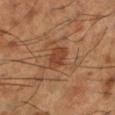A male subject, approximately 65 years of age. A 15 mm crop from a total-body photograph taken for skin-cancer surveillance. Approximately 3 mm at its widest. On the left lower leg.